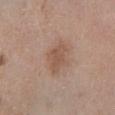Part of a total-body skin-imaging series; this lesion was reviewed on a skin check and was not flagged for biopsy.
A female patient, in their mid- to late 70s.
Located on the left lower leg.
This image is a 15 mm lesion crop taken from a total-body photograph.